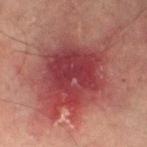| feature | finding |
|---|---|
| follow-up | imaged on a skin check; not biopsied |
| site | the left thigh |
| image | 15 mm crop, total-body photography |
| patient | male, aged 73–77 |